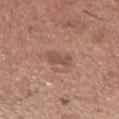Findings:
• site — the head or neck
• diameter — ≈3 mm
• acquisition — total-body-photography crop, ~15 mm field of view
• image-analysis metrics — a within-lesion color-variation index near 1/10 and peripheral color asymmetry of about 0.5; a nevus-likeness score of about 0/100 and lesion-presence confidence of about 100/100
• patient — male, roughly 65 years of age
• lighting — white-light